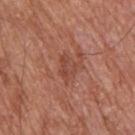<lesion>
<biopsy_status>not biopsied; imaged during a skin examination</biopsy_status>
<automated_metrics>
  <area_mm2_approx>4.5</area_mm2_approx>
  <shape_asymmetry>0.3</shape_asymmetry>
  <cielab_L>46</cielab_L>
  <cielab_a>26</cielab_a>
  <cielab_b>29</cielab_b>
  <vs_skin_contrast_norm>5.5</vs_skin_contrast_norm>
  <border_irregularity_0_10>3.0</border_irregularity_0_10>
  <color_variation_0_10>2.0</color_variation_0_10>
  <peripheral_color_asymmetry>0.5</peripheral_color_asymmetry>
</automated_metrics>
<patient>
  <sex>male</sex>
  <age_approx>65</age_approx>
</patient>
<lighting>white-light</lighting>
<image>
  <source>total-body photography crop</source>
  <field_of_view_mm>15</field_of_view_mm>
</image>
<site>upper back</site>
</lesion>